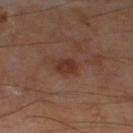Impression:
This lesion was catalogued during total-body skin photography and was not selected for biopsy.
Context:
A 15 mm close-up tile from a total-body photography series done for melanoma screening. Imaged with cross-polarized lighting. A male patient in their mid- to late 60s. An algorithmic analysis of the crop reported internal color variation of about 2.5 on a 0–10 scale and a peripheral color-asymmetry measure near 1. The analysis additionally found an automated nevus-likeness rating near 75 out of 100 and a detector confidence of about 100 out of 100 that the crop contains a lesion. About 3 mm across. From the leg.